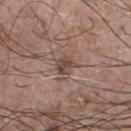Captured during whole-body skin photography for melanoma surveillance; the lesion was not biopsied. This image is a 15 mm lesion crop taken from a total-body photograph. The lesion is located on the chest. The lesion's longest dimension is about 2.5 mm. A male subject, aged around 75.